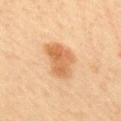{
  "biopsy_status": "not biopsied; imaged during a skin examination",
  "image": {
    "source": "total-body photography crop",
    "field_of_view_mm": 15
  },
  "site": "upper back",
  "lesion_size": {
    "long_diameter_mm_approx": 4.5
  },
  "automated_metrics": {
    "area_mm2_approx": 12.0,
    "eccentricity": 0.7,
    "shape_asymmetry": 0.3,
    "cielab_L": 56,
    "cielab_a": 20,
    "cielab_b": 36,
    "vs_skin_darker_L": 10.0,
    "vs_skin_contrast_norm": 7.5,
    "border_irregularity_0_10": 3.0,
    "peripheral_color_asymmetry": 1.0
  },
  "patient": {
    "sex": "female",
    "age_approx": 45
  }
}